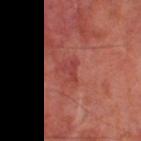{
  "biopsy_status": "not biopsied; imaged during a skin examination",
  "site": "leg",
  "automated_metrics": {
    "area_mm2_approx": 2.5,
    "shape_asymmetry": 0.7,
    "nevus_likeness_0_100": 0,
    "lesion_detection_confidence_0_100": 90
  },
  "lesion_size": {
    "long_diameter_mm_approx": 2.5
  },
  "lighting": "cross-polarized",
  "patient": {
    "sex": "male",
    "age_approx": 70
  },
  "image": {
    "source": "total-body photography crop",
    "field_of_view_mm": 15
  }
}